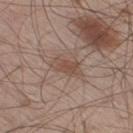Case summary:
- lighting: white-light
- size: ~4 mm (longest diameter)
- automated lesion analysis: a lesion area of about 6 mm² and two-axis asymmetry of about 0.3; a border-irregularity rating of about 3/10 and a color-variation rating of about 3/10; a nevus-likeness score of about 0/100
- imaging modality: ~15 mm crop, total-body skin-cancer survey
- body site: the right thigh
- subject: male, about 60 years old